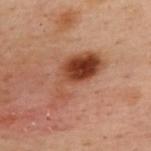The lesion was tiled from a total-body skin photograph and was not biopsied. Cropped from a total-body skin-imaging series; the visible field is about 15 mm. The patient is a female about 55 years old. The lesion is on the upper back.